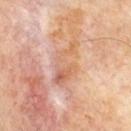No biopsy was performed on this lesion — it was imaged during a full skin examination and was not determined to be concerning.
A 15 mm close-up tile from a total-body photography series done for melanoma screening.
Approximately 6 mm at its widest.
The tile uses cross-polarized illumination.
The lesion-visualizer software estimated an area of roughly 11 mm² and a symmetry-axis asymmetry near 0.45. The software also gave a within-lesion color-variation index near 6/10.
Located on the mid back.
A male subject, about 60 years old.